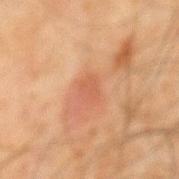The lesion was photographed on a routine skin check and not biopsied; there is no pathology result. A lesion tile, about 15 mm wide, cut from a 3D total-body photograph. A male subject approximately 65 years of age. Approximately 2.5 mm at its widest. Imaged with cross-polarized lighting. On the mid back. Automated image analysis of the tile measured a footprint of about 4 mm² and a shape-asymmetry score of about 0.25 (0 = symmetric). And it measured lesion-presence confidence of about 100/100.